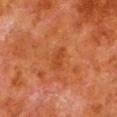Impression:
This lesion was catalogued during total-body skin photography and was not selected for biopsy.
Acquisition and patient details:
A roughly 15 mm field-of-view crop from a total-body skin photograph. The lesion is on the left lower leg. The recorded lesion diameter is about 3 mm. A male subject roughly 80 years of age. Captured under cross-polarized illumination. The total-body-photography lesion software estimated a lesion area of about 3 mm², an eccentricity of roughly 0.9, and a shape-asymmetry score of about 0.3 (0 = symmetric). It also reported a lesion color around L≈35 a*≈26 b*≈34 in CIELAB and a lesion-to-skin contrast of about 5 (normalized; higher = more distinct).